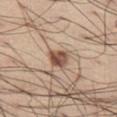Recorded during total-body skin imaging; not selected for excision or biopsy.
A male patient in their mid- to late 50s.
Automated image analysis of the tile measured a footprint of about 5 mm², an eccentricity of roughly 0.4, and a symmetry-axis asymmetry near 0.25.
About 2.5 mm across.
Imaged with white-light lighting.
Cropped from a total-body skin-imaging series; the visible field is about 15 mm.
The lesion is on the left thigh.